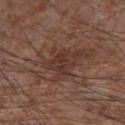notes: imaged on a skin check; not biopsied
subject: male, aged 73–77
site: the right forearm
automated lesion analysis: a border-irregularity rating of about 6.5/10 and a within-lesion color-variation index near 5/10; an automated nevus-likeness rating near 0 out of 100 and a lesion-detection confidence of about 100/100
diameter: about 8 mm
image: ~15 mm crop, total-body skin-cancer survey
illumination: white-light illumination